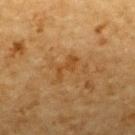Q: Was this lesion biopsied?
A: imaged on a skin check; not biopsied
Q: Automated lesion metrics?
A: a lesion area of about 3 mm², a shape eccentricity near 0.9, and two-axis asymmetry of about 0.6; border irregularity of about 7.5 on a 0–10 scale and a color-variation rating of about 0/10; an automated nevus-likeness rating near 0 out of 100 and a lesion-detection confidence of about 100/100
Q: What are the patient's age and sex?
A: male, in their mid-80s or older
Q: What kind of image is this?
A: 15 mm crop, total-body photography
Q: How large is the lesion?
A: about 3 mm
Q: Lesion location?
A: the upper back
Q: Illumination type?
A: cross-polarized